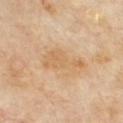{
  "biopsy_status": "not biopsied; imaged during a skin examination",
  "site": "front of the torso",
  "patient": {
    "sex": "male",
    "age_approx": 70
  },
  "lighting": "cross-polarized",
  "lesion_size": {
    "long_diameter_mm_approx": 5.5
  },
  "image": {
    "source": "total-body photography crop",
    "field_of_view_mm": 15
  }
}